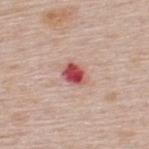  biopsy_status: not biopsied; imaged during a skin examination
  patient:
    sex: male
    age_approx: 60
  site: upper back
  automated_metrics:
    area_mm2_approx: 4.5
    shape_asymmetry: 0.2
    cielab_L: 51
    cielab_a: 34
    cielab_b: 25
    vs_skin_contrast_norm: 11.5
  image:
    source: total-body photography crop
    field_of_view_mm: 15
  lesion_size:
    long_diameter_mm_approx: 2.5